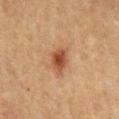| feature | finding |
|---|---|
| follow-up | imaged on a skin check; not biopsied |
| size | ≈3.5 mm |
| body site | the chest |
| illumination | cross-polarized illumination |
| automated lesion analysis | a border-irregularity rating of about 2/10 and internal color variation of about 3 on a 0–10 scale; a lesion-detection confidence of about 100/100 |
| acquisition | ~15 mm crop, total-body skin-cancer survey |
| subject | male, approximately 50 years of age |